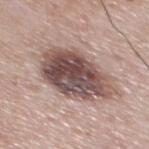Recorded during total-body skin imaging; not selected for excision or biopsy. A 15 mm crop from a total-body photograph taken for skin-cancer surveillance. Measured at roughly 7.5 mm in maximum diameter. A male subject, approximately 65 years of age. This is a white-light tile. From the back.